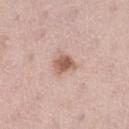Case summary:
– biopsy status: total-body-photography surveillance lesion; no biopsy
– imaging modality: ~15 mm tile from a whole-body skin photo
– site: the left lower leg
– illumination: white-light illumination
– automated lesion analysis: an eccentricity of roughly 0.6 and a symmetry-axis asymmetry near 0.3; an automated nevus-likeness rating near 75 out of 100 and a detector confidence of about 100 out of 100 that the crop contains a lesion
– subject: female, approximately 40 years of age